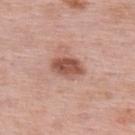The tile uses white-light illumination.
This image is a 15 mm lesion crop taken from a total-body photograph.
On the upper back.
A female subject approximately 50 years of age.